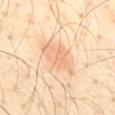<case>
  <biopsy_status>not biopsied; imaged during a skin examination</biopsy_status>
  <site>mid back</site>
  <lighting>cross-polarized</lighting>
  <patient>
    <sex>male</sex>
    <age_approx>40</age_approx>
  </patient>
  <image>
    <source>total-body photography crop</source>
    <field_of_view_mm>15</field_of_view_mm>
  </image>
  <automated_metrics>
    <area_mm2_approx>11.0</area_mm2_approx>
    <eccentricity>0.85</eccentricity>
    <vs_skin_darker_L>8.0</vs_skin_darker_L>
    <vs_skin_contrast_norm>5.0</vs_skin_contrast_norm>
  </automated_metrics>
</case>